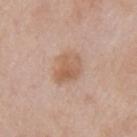Q: Is there a histopathology result?
A: no biopsy performed (imaged during a skin exam)
Q: Patient demographics?
A: female, aged 38 to 42
Q: What is the imaging modality?
A: ~15 mm crop, total-body skin-cancer survey
Q: How large is the lesion?
A: about 4 mm
Q: What did automated image analysis measure?
A: border irregularity of about 2.5 on a 0–10 scale and a peripheral color-asymmetry measure near 1.5
Q: Where on the body is the lesion?
A: the left upper arm
Q: Illumination type?
A: white-light illumination